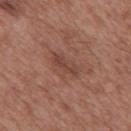The lesion was photographed on a routine skin check and not biopsied; there is no pathology result. Located on the mid back. Cropped from a whole-body photographic skin survey; the tile spans about 15 mm. The lesion's longest dimension is about 3.5 mm. The tile uses white-light illumination. The subject is a male aged approximately 50.